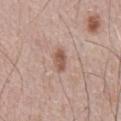  biopsy_status: not biopsied; imaged during a skin examination